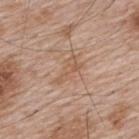Clinical impression:
The lesion was tiled from a total-body skin photograph and was not biopsied.
Clinical summary:
A male subject approximately 65 years of age. This is a white-light tile. A region of skin cropped from a whole-body photographic capture, roughly 15 mm wide. The lesion's longest dimension is about 4 mm. Located on the upper back.